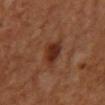Background:
A lesion tile, about 15 mm wide, cut from a 3D total-body photograph. The lesion is on the chest. A male patient aged 58–62.